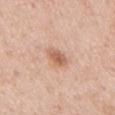biopsy status: total-body-photography surveillance lesion; no biopsy
lesion diameter: about 3 mm
illumination: white-light illumination
subject: male, roughly 55 years of age
imaging modality: ~15 mm crop, total-body skin-cancer survey
site: the mid back
TBP lesion metrics: a lesion area of about 5 mm², an outline eccentricity of about 0.7 (0 = round, 1 = elongated), and a symmetry-axis asymmetry near 0.25; a lesion color around L≈62 a*≈21 b*≈32 in CIELAB and a normalized lesion–skin contrast near 7.5; a border-irregularity index near 2/10, internal color variation of about 4 on a 0–10 scale, and a peripheral color-asymmetry measure near 1.5; a nevus-likeness score of about 75/100 and a lesion-detection confidence of about 100/100